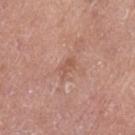This lesion was catalogued during total-body skin photography and was not selected for biopsy. Captured under white-light illumination. The lesion is on the left thigh. The patient is a female aged 63 to 67. Automated tile analysis of the lesion measured a lesion area of about 3 mm², an eccentricity of roughly 0.9, and two-axis asymmetry of about 0.35. The software also gave border irregularity of about 3 on a 0–10 scale. A region of skin cropped from a whole-body photographic capture, roughly 15 mm wide.